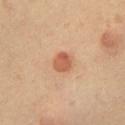Part of a total-body skin-imaging series; this lesion was reviewed on a skin check and was not flagged for biopsy. From the chest. Imaged with cross-polarized lighting. The subject is a male aged 58 to 62. Approximately 2.5 mm at its widest. A 15 mm close-up tile from a total-body photography series done for melanoma screening.